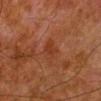workup: total-body-photography surveillance lesion; no biopsy | body site: the left lower leg | imaging modality: 15 mm crop, total-body photography | lighting: cross-polarized illumination | subject: male, roughly 80 years of age.